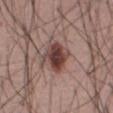Imaged during a routine full-body skin examination; the lesion was not biopsied and no histopathology is available.
A 15 mm close-up tile from a total-body photography series done for melanoma screening.
Automated image analysis of the tile measured an average lesion color of about L≈41 a*≈20 b*≈21 (CIELAB), about 15 CIELAB-L* units darker than the surrounding skin, and a normalized border contrast of about 11. The software also gave a border-irregularity index near 2/10, a within-lesion color-variation index near 5.5/10, and a peripheral color-asymmetry measure near 1.5.
The lesion is located on the abdomen.
The recorded lesion diameter is about 4 mm.
A male subject about 55 years old.